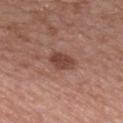No biopsy was performed on this lesion — it was imaged during a full skin examination and was not determined to be concerning. About 3.5 mm across. Imaged with white-light lighting. A 15 mm crop from a total-body photograph taken for skin-cancer surveillance. Located on the mid back. The patient is a male aged 63 to 67.